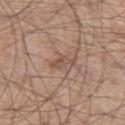<tbp_lesion>
  <biopsy_status>not biopsied; imaged during a skin examination</biopsy_status>
  <lesion_size>
    <long_diameter_mm_approx>3.0</long_diameter_mm_approx>
  </lesion_size>
  <image>
    <source>total-body photography crop</source>
    <field_of_view_mm>15</field_of_view_mm>
  </image>
  <patient>
    <sex>male</sex>
    <age_approx>45</age_approx>
  </patient>
  <automated_metrics>
    <eccentricity>0.9</eccentricity>
    <shape_asymmetry>0.5</shape_asymmetry>
    <cielab_L>51</cielab_L>
    <cielab_a>19</cielab_a>
    <cielab_b>26</cielab_b>
    <vs_skin_darker_L>8.0</vs_skin_darker_L>
    <vs_skin_contrast_norm>6.0</vs_skin_contrast_norm>
    <border_irregularity_0_10>5.5</border_irregularity_0_10>
    <color_variation_0_10>0.0</color_variation_0_10>
  </automated_metrics>
  <site>left thigh</site>
</tbp_lesion>